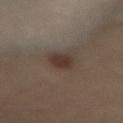A roughly 15 mm field-of-view crop from a total-body skin photograph.
The patient is a female in their 60s.
Approximately 3 mm at its widest.
From the back.